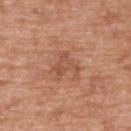Findings:
* follow-up — catalogued during a skin exam; not biopsied
* subject — male, about 50 years old
* lesion size — about 3.5 mm
* acquisition — ~15 mm crop, total-body skin-cancer survey
* site — the upper back